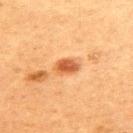Q: Is there a histopathology result?
A: total-body-photography surveillance lesion; no biopsy
Q: What are the patient's age and sex?
A: female, aged around 60
Q: How was the tile lit?
A: cross-polarized
Q: What is the anatomic site?
A: the upper back
Q: What kind of image is this?
A: ~15 mm crop, total-body skin-cancer survey